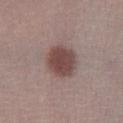Impression: No biopsy was performed on this lesion — it was imaged during a full skin examination and was not determined to be concerning. Image and clinical context: The total-body-photography lesion software estimated a mean CIELAB color near L≈46 a*≈18 b*≈20, roughly 12 lightness units darker than nearby skin, and a normalized border contrast of about 9. It also reported a border-irregularity index near 1.5/10 and peripheral color asymmetry of about 1. Measured at roughly 4 mm in maximum diameter. A 15 mm crop from a total-body photograph taken for skin-cancer surveillance. The patient is a female roughly 20 years of age. The lesion is located on the right lower leg.